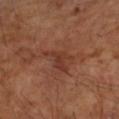Acquisition and patient details: An algorithmic analysis of the crop reported a lesion area of about 6.5 mm² and a symmetry-axis asymmetry near 0.6. The patient is a male aged approximately 65. The lesion's longest dimension is about 4 mm. A lesion tile, about 15 mm wide, cut from a 3D total-body photograph. On the right upper arm. Captured under cross-polarized illumination.